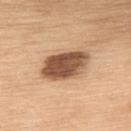follow-up: no biopsy performed (imaged during a skin exam)
lighting: white-light
diameter: ≈6 mm
image source: total-body-photography crop, ~15 mm field of view
body site: the upper back
subject: female, aged approximately 55